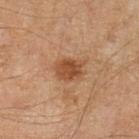Q: Is there a histopathology result?
A: catalogued during a skin exam; not biopsied
Q: What lighting was used for the tile?
A: cross-polarized illumination
Q: Automated lesion metrics?
A: internal color variation of about 3 on a 0–10 scale and peripheral color asymmetry of about 1; a nevus-likeness score of about 85/100 and a lesion-detection confidence of about 100/100
Q: Lesion size?
A: ~3.5 mm (longest diameter)
Q: What is the anatomic site?
A: the left lower leg
Q: How was this image acquired?
A: 15 mm crop, total-body photography
Q: Who is the patient?
A: aged approximately 55